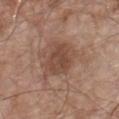Assessment: The lesion was photographed on a routine skin check and not biopsied; there is no pathology result. Acquisition and patient details: The patient is a male aged approximately 65. About 5 mm across. The tile uses white-light illumination. On the chest. The lesion-visualizer software estimated a mean CIELAB color near L≈47 a*≈19 b*≈27 and roughly 9 lightness units darker than nearby skin. A 15 mm crop from a total-body photograph taken for skin-cancer surveillance.